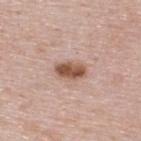The lesion was photographed on a routine skin check and not biopsied; there is no pathology result. This is a white-light tile. Automated image analysis of the tile measured a border-irregularity rating of about 2.5/10 and a within-lesion color-variation index near 3.5/10. The software also gave a lesion-detection confidence of about 100/100. Cropped from a total-body skin-imaging series; the visible field is about 15 mm. The recorded lesion diameter is about 3.5 mm. A female subject, aged approximately 50. From the upper back.